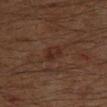Cropped from a whole-body photographic skin survey; the tile spans about 15 mm.
About 2.5 mm across.
The subject is a male aged around 60.
Located on the right lower leg.
The tile uses cross-polarized illumination.